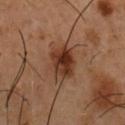<lesion>
  <biopsy_status>not biopsied; imaged during a skin examination</biopsy_status>
  <site>chest</site>
  <image>
    <source>total-body photography crop</source>
    <field_of_view_mm>15</field_of_view_mm>
  </image>
  <patient>
    <sex>male</sex>
    <age_approx>55</age_approx>
  </patient>
</lesion>